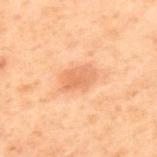The lesion was photographed on a routine skin check and not biopsied; there is no pathology result.